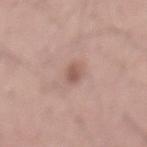Captured during whole-body skin photography for melanoma surveillance; the lesion was not biopsied. A male subject, aged approximately 55. The lesion is located on the front of the torso. A region of skin cropped from a whole-body photographic capture, roughly 15 mm wide.